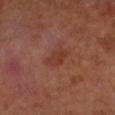notes = no biopsy performed (imaged during a skin exam) | location = the left upper arm | tile lighting = cross-polarized illumination | image = ~15 mm tile from a whole-body skin photo | automated lesion analysis = an area of roughly 5 mm², an eccentricity of roughly 0.8, and two-axis asymmetry of about 0.3; a border-irregularity rating of about 3/10; an automated nevus-likeness rating near 0 out of 100 and a detector confidence of about 100 out of 100 that the crop contains a lesion | lesion diameter = ~3 mm (longest diameter) | patient = male, in their mid-50s.